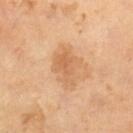Context:
Imaged with cross-polarized lighting. The lesion's longest dimension is about 4 mm. A lesion tile, about 15 mm wide, cut from a 3D total-body photograph. A male patient, in their mid- to late 60s. Automated image analysis of the tile measured a mean CIELAB color near L≈61 a*≈21 b*≈38 and about 8 CIELAB-L* units darker than the surrounding skin.